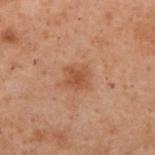Assessment: No biopsy was performed on this lesion — it was imaged during a full skin examination and was not determined to be concerning. Image and clinical context: A female subject, in their mid-50s. The lesion is on the upper back. The lesion-visualizer software estimated an average lesion color of about L≈42 a*≈20 b*≈29 (CIELAB), about 7 CIELAB-L* units darker than the surrounding skin, and a normalized border contrast of about 6.5. It also reported a nevus-likeness score of about 25/100 and a lesion-detection confidence of about 100/100. This image is a 15 mm lesion crop taken from a total-body photograph. The recorded lesion diameter is about 2.5 mm.